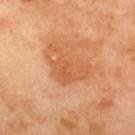| field | value |
|---|---|
| follow-up | imaged on a skin check; not biopsied |
| patient | male, roughly 70 years of age |
| imaging modality | ~15 mm tile from a whole-body skin photo |
| site | the upper back |
| image-analysis metrics | an area of roughly 12 mm² and a shape eccentricity near 0.75; a mean CIELAB color near L≈57 a*≈26 b*≈41 and a normalized lesion–skin contrast near 5; a border-irregularity rating of about 6.5/10, internal color variation of about 3 on a 0–10 scale, and a peripheral color-asymmetry measure near 1 |
| illumination | cross-polarized illumination |
| lesion size | ≈5.5 mm |